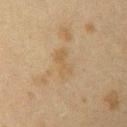Q: Was a biopsy performed?
A: catalogued during a skin exam; not biopsied
Q: What lighting was used for the tile?
A: cross-polarized
Q: What are the patient's age and sex?
A: female, approximately 40 years of age
Q: What is the anatomic site?
A: the left upper arm
Q: How large is the lesion?
A: about 4 mm
Q: How was this image acquired?
A: ~15 mm crop, total-body skin-cancer survey